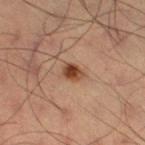No biopsy was performed on this lesion — it was imaged during a full skin examination and was not determined to be concerning. A male subject, approximately 35 years of age. A close-up tile cropped from a whole-body skin photograph, about 15 mm across. The recorded lesion diameter is about 2.5 mm. Located on the right thigh. The tile uses cross-polarized illumination.